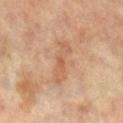follow-up: no biopsy performed (imaged during a skin exam)
image source: ~15 mm tile from a whole-body skin photo
subject: female, in their mid- to late 60s
automated metrics: a lesion area of about 7 mm², an eccentricity of roughly 0.95, and a symmetry-axis asymmetry near 0.3
lighting: cross-polarized
location: the left leg
size: about 5 mm